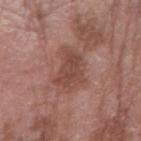Part of a total-body skin-imaging series; this lesion was reviewed on a skin check and was not flagged for biopsy. This is a white-light tile. Located on the right forearm. A male subject roughly 75 years of age. A region of skin cropped from a whole-body photographic capture, roughly 15 mm wide. Longest diameter approximately 4.5 mm.